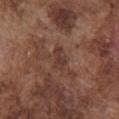  biopsy_status: not biopsied; imaged during a skin examination
  image:
    source: total-body photography crop
    field_of_view_mm: 15
  patient:
    sex: male
    age_approx: 75
  lesion_size:
    long_diameter_mm_approx: 2.5
  lighting: white-light
  automated_metrics:
    nevus_likeness_0_100: 0
    lesion_detection_confidence_0_100: 100
  site: chest